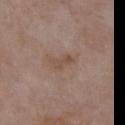<tbp_lesion>
  <biopsy_status>not biopsied; imaged during a skin examination</biopsy_status>
  <lighting>white-light</lighting>
  <image>
    <source>total-body photography crop</source>
    <field_of_view_mm>15</field_of_view_mm>
  </image>
  <lesion_size>
    <long_diameter_mm_approx>2.5</long_diameter_mm_approx>
  </lesion_size>
  <site>front of the torso</site>
  <patient>
    <sex>female</sex>
    <age_approx>85+</age_approx>
  </patient>
</tbp_lesion>